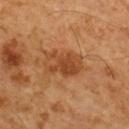{
  "patient": {
    "sex": "male",
    "age_approx": 60
  },
  "image": {
    "source": "total-body photography crop",
    "field_of_view_mm": 15
  },
  "site": "upper back",
  "lighting": "cross-polarized",
  "lesion_size": {
    "long_diameter_mm_approx": 4.5
  },
  "automated_metrics": {
    "area_mm2_approx": 9.5,
    "shape_asymmetry": 0.35
  }
}